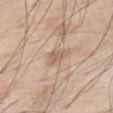No biopsy was performed on this lesion — it was imaged during a full skin examination and was not determined to be concerning.
Captured under white-light illumination.
From the right thigh.
Measured at roughly 3 mm in maximum diameter.
A male patient, about 70 years old.
A 15 mm crop from a total-body photograph taken for skin-cancer surveillance.